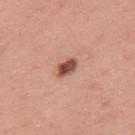The lesion was photographed on a routine skin check and not biopsied; there is no pathology result.
A male subject, aged 38–42.
A 15 mm close-up extracted from a 3D total-body photography capture.
From the upper back.
Automated tile analysis of the lesion measured a border-irregularity rating of about 2/10, a within-lesion color-variation index near 4/10, and a peripheral color-asymmetry measure near 1.5. And it measured a classifier nevus-likeness of about 95/100 and lesion-presence confidence of about 100/100.